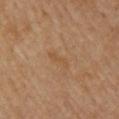{"biopsy_status": "not biopsied; imaged during a skin examination", "image": {"source": "total-body photography crop", "field_of_view_mm": 15}, "patient": {"sex": "male", "age_approx": "85+"}, "automated_metrics": {"area_mm2_approx": 2.5, "shape_asymmetry": 0.45, "cielab_L": 49, "cielab_a": 18, "cielab_b": 34, "vs_skin_darker_L": 5.0, "border_irregularity_0_10": 5.5}, "site": "upper back"}